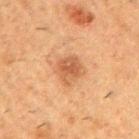Impression: Recorded during total-body skin imaging; not selected for excision or biopsy. Image and clinical context: The total-body-photography lesion software estimated a classifier nevus-likeness of about 55/100 and lesion-presence confidence of about 100/100. Captured under cross-polarized illumination. The patient is a male approximately 50 years of age. About 3 mm across. A lesion tile, about 15 mm wide, cut from a 3D total-body photograph. From the right upper arm.